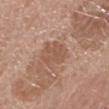The tile uses white-light illumination.
On the right forearm.
A 15 mm crop from a total-body photograph taken for skin-cancer surveillance.
The recorded lesion diameter is about 3 mm.
The subject is a female about 55 years old.
Automated image analysis of the tile measured a footprint of about 6.5 mm² and a symmetry-axis asymmetry near 0.3. It also reported an average lesion color of about L≈53 a*≈20 b*≈29 (CIELAB), a lesion–skin lightness drop of about 6, and a normalized lesion–skin contrast near 5. It also reported lesion-presence confidence of about 100/100.